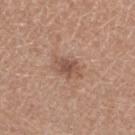This lesion was catalogued during total-body skin photography and was not selected for biopsy. A female patient, approximately 35 years of age. A 15 mm close-up tile from a total-body photography series done for melanoma screening. The tile uses white-light illumination. The recorded lesion diameter is about 3 mm. From the leg.